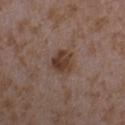<tbp_lesion>
<biopsy_status>not biopsied; imaged during a skin examination</biopsy_status>
<image>
  <source>total-body photography crop</source>
  <field_of_view_mm>15</field_of_view_mm>
</image>
<patient>
  <sex>female</sex>
  <age_approx>35</age_approx>
</patient>
<site>left forearm</site>
<lighting>white-light</lighting>
<automated_metrics>
  <color_variation_0_10>4.5</color_variation_0_10>
  <lesion_detection_confidence_0_100>100</lesion_detection_confidence_0_100>
</automated_metrics>
<lesion_size>
  <long_diameter_mm_approx>3.0</long_diameter_mm_approx>
</lesion_size>
</tbp_lesion>